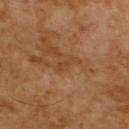notes = imaged on a skin check; not biopsied | subject = male, aged around 65 | site = the upper back | size = ~2.5 mm (longest diameter) | acquisition = ~15 mm crop, total-body skin-cancer survey.